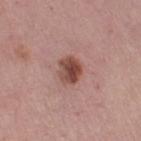biopsy status=total-body-photography surveillance lesion; no biopsy
acquisition=total-body-photography crop, ~15 mm field of view
anatomic site=the left thigh
lesion diameter=~3 mm (longest diameter)
subject=female, approximately 65 years of age